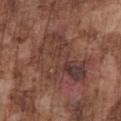Image and clinical context:
Located on the chest. The recorded lesion diameter is about 8 mm. The lesion-visualizer software estimated an area of roughly 34 mm², a shape eccentricity near 0.7, and two-axis asymmetry of about 0.35. It also reported a lesion-detection confidence of about 95/100. Captured under white-light illumination. The patient is a male aged 73 to 77. A close-up tile cropped from a whole-body skin photograph, about 15 mm across.